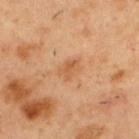Findings:
• follow-up — no biopsy performed (imaged during a skin exam)
• image source — ~15 mm tile from a whole-body skin photo
• body site — the upper back
• tile lighting — cross-polarized illumination
• subject — male, about 55 years old
• automated lesion analysis — an area of roughly 4 mm² and a shape eccentricity near 0.7; a border-irregularity index near 3.5/10, a color-variation rating of about 2/10, and a peripheral color-asymmetry measure near 0.5; an automated nevus-likeness rating near 30 out of 100 and a detector confidence of about 100 out of 100 that the crop contains a lesion
• lesion size — ≈3 mm